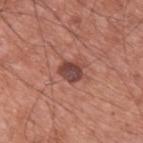| field | value |
|---|---|
| image source | ~15 mm crop, total-body skin-cancer survey |
| size | ~3 mm (longest diameter) |
| tile lighting | white-light illumination |
| anatomic site | the upper back |
| subject | male, approximately 60 years of age |